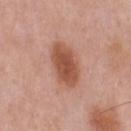Impression: No biopsy was performed on this lesion — it was imaged during a full skin examination and was not determined to be concerning. Background: A male patient aged 53–57. Approximately 5.5 mm at its widest. This image is a 15 mm lesion crop taken from a total-body photograph. From the chest.